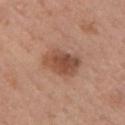notes: imaged on a skin check; not biopsied | patient: female, aged around 50 | imaging modality: ~15 mm crop, total-body skin-cancer survey | tile lighting: white-light illumination | size: ~4.5 mm (longest diameter) | site: the left upper arm.